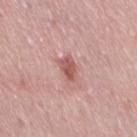{
  "biopsy_status": "not biopsied; imaged during a skin examination",
  "patient": {
    "sex": "female",
    "age_approx": 40
  },
  "image": {
    "source": "total-body photography crop",
    "field_of_view_mm": 15
  },
  "site": "right thigh"
}